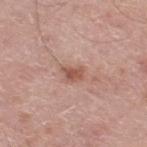Clinical impression:
The lesion was photographed on a routine skin check and not biopsied; there is no pathology result.
Background:
A 15 mm crop from a total-body photograph taken for skin-cancer surveillance. The subject is a male aged 78 to 82. The tile uses white-light illumination. Located on the right thigh.